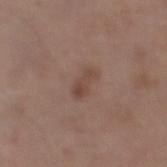Clinical impression: This lesion was catalogued during total-body skin photography and was not selected for biopsy. Context: Captured under white-light illumination. This image is a 15 mm lesion crop taken from a total-body photograph. The patient is a male roughly 65 years of age. About 3 mm across. Located on the right lower leg. An algorithmic analysis of the crop reported an average lesion color of about L≈45 a*≈18 b*≈24 (CIELAB), roughly 7 lightness units darker than nearby skin, and a lesion-to-skin contrast of about 6 (normalized; higher = more distinct). The analysis additionally found a border-irregularity index near 4/10 and internal color variation of about 1.5 on a 0–10 scale.